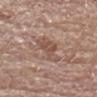biopsy status = imaged on a skin check; not biopsied
body site = the chest
patient = male, in their 80s
illumination = white-light illumination
TBP lesion metrics = a footprint of about 8 mm², a shape eccentricity near 0.85, and a symmetry-axis asymmetry near 0.45; a border-irregularity index near 4.5/10, a within-lesion color-variation index near 2.5/10, and radial color variation of about 0.5; a classifier nevus-likeness of about 0/100
image = total-body-photography crop, ~15 mm field of view